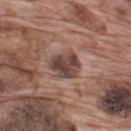biopsy_status: not biopsied; imaged during a skin examination
lighting: white-light
lesion_size:
  long_diameter_mm_approx: 4.0
automated_metrics:
  area_mm2_approx: 9.5
  eccentricity: 0.7
  shape_asymmetry: 0.2
  cielab_L: 43
  cielab_a: 18
  cielab_b: 21
  vs_skin_darker_L: 12.0
  vs_skin_contrast_norm: 10.0
  lesion_detection_confidence_0_100: 100
patient:
  sex: male
  age_approx: 70
site: upper back
image:
  source: total-body photography crop
  field_of_view_mm: 15